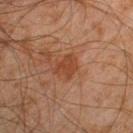Q: Was this lesion biopsied?
A: no biopsy performed (imaged during a skin exam)
Q: Who is the patient?
A: male, roughly 45 years of age
Q: What is the lesion's diameter?
A: ~3 mm (longest diameter)
Q: What did automated image analysis measure?
A: a shape eccentricity near 0.45 and two-axis asymmetry of about 0.45; border irregularity of about 5 on a 0–10 scale, a within-lesion color-variation index near 1.5/10, and radial color variation of about 0.5
Q: What kind of image is this?
A: ~15 mm tile from a whole-body skin photo
Q: What lighting was used for the tile?
A: cross-polarized illumination
Q: What is the anatomic site?
A: the right lower leg